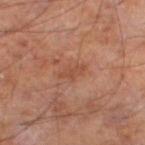The lesion was photographed on a routine skin check and not biopsied; there is no pathology result.
A male subject aged around 55.
From the leg.
Captured under cross-polarized illumination.
A lesion tile, about 15 mm wide, cut from a 3D total-body photograph.